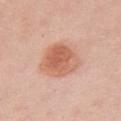biopsy status = no biopsy performed (imaged during a skin exam) | lesion size = about 4.5 mm | image source = ~15 mm tile from a whole-body skin photo | image-analysis metrics = roughly 11 lightness units darker than nearby skin and a normalized border contrast of about 7.5 | illumination = white-light illumination | patient = female, aged around 50 | site = the chest.